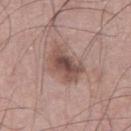Case summary:
• notes — imaged on a skin check; not biopsied
• anatomic site — the leg
• tile lighting — white-light
• imaging modality — total-body-photography crop, ~15 mm field of view
• lesion size — ~5 mm (longest diameter)
• patient — male, about 75 years old